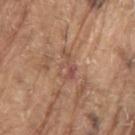notes=total-body-photography surveillance lesion; no biopsy
lesion size=about 3.5 mm
patient=male, roughly 80 years of age
body site=the arm
acquisition=total-body-photography crop, ~15 mm field of view